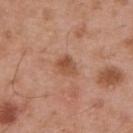This lesion was catalogued during total-body skin photography and was not selected for biopsy.
The lesion is on the upper back.
Measured at roughly 2.5 mm in maximum diameter.
The tile uses white-light illumination.
The lesion-visualizer software estimated a border-irregularity index near 2.5/10. The analysis additionally found a classifier nevus-likeness of about 75/100 and a detector confidence of about 100 out of 100 that the crop contains a lesion.
A close-up tile cropped from a whole-body skin photograph, about 15 mm across.
The subject is a male aged around 55.